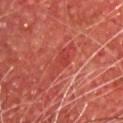No biopsy was performed on this lesion — it was imaged during a full skin examination and was not determined to be concerning. This is a cross-polarized tile. About 5 mm across. Automated image analysis of the tile measured a footprint of about 6 mm² and a shape eccentricity near 0.95. And it measured a nevus-likeness score of about 0/100 and a lesion-detection confidence of about 70/100. A 15 mm close-up extracted from a 3D total-body photography capture. A male subject, aged 63–67. Located on the front of the torso.